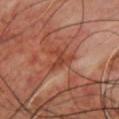biopsy status: imaged on a skin check; not biopsied | tile lighting: cross-polarized illumination | automated metrics: a lesion area of about 4.5 mm² and two-axis asymmetry of about 0.65; an average lesion color of about L≈40 a*≈28 b*≈31 (CIELAB), a lesion–skin lightness drop of about 8, and a normalized lesion–skin contrast near 7; a color-variation rating of about 1/10 and radial color variation of about 0.5 | acquisition: total-body-photography crop, ~15 mm field of view | site: the chest | subject: male, in their mid- to late 40s.